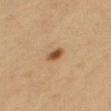* follow-up: no biopsy performed (imaged during a skin exam)
* illumination: cross-polarized
* patient: female, roughly 55 years of age
* automated metrics: an area of roughly 3.5 mm² and two-axis asymmetry of about 0.2; a mean CIELAB color near L≈44 a*≈18 b*≈31, about 12 CIELAB-L* units darker than the surrounding skin, and a lesion-to-skin contrast of about 9.5 (normalized; higher = more distinct); a nevus-likeness score of about 100/100
* lesion diameter: ~2.5 mm (longest diameter)
* location: the leg
* image source: 15 mm crop, total-body photography